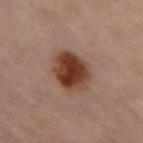Impression:
The lesion was tiled from a total-body skin photograph and was not biopsied.
Context:
The lesion is located on the right leg. The patient is a female approximately 60 years of age. Captured under cross-polarized illumination. Measured at roughly 4.5 mm in maximum diameter. A 15 mm close-up tile from a total-body photography series done for melanoma screening. Automated tile analysis of the lesion measured a lesion area of about 13 mm², an outline eccentricity of about 0.55 (0 = round, 1 = elongated), and two-axis asymmetry of about 0.1. It also reported an average lesion color of about L≈33 a*≈20 b*≈25 (CIELAB), roughly 13 lightness units darker than nearby skin, and a normalized lesion–skin contrast near 12.5. The software also gave border irregularity of about 1.5 on a 0–10 scale and internal color variation of about 5 on a 0–10 scale. And it measured an automated nevus-likeness rating near 100 out of 100 and a detector confidence of about 100 out of 100 that the crop contains a lesion.